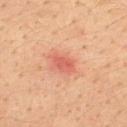No biopsy was performed on this lesion — it was imaged during a full skin examination and was not determined to be concerning.
The patient is a male approximately 35 years of age.
A 15 mm close-up tile from a total-body photography series done for melanoma screening.
On the upper back.
The recorded lesion diameter is about 3 mm.